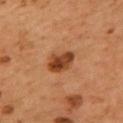Recorded during total-body skin imaging; not selected for excision or biopsy.
A male subject aged around 55.
A lesion tile, about 15 mm wide, cut from a 3D total-body photograph.
An algorithmic analysis of the crop reported a shape eccentricity near 0.7. The analysis additionally found a lesion–skin lightness drop of about 13 and a normalized border contrast of about 10. And it measured a border-irregularity rating of about 1.5/10 and a color-variation rating of about 5/10.
Imaged with cross-polarized lighting.
On the mid back.
About 3.5 mm across.